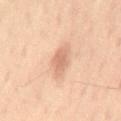Recorded during total-body skin imaging; not selected for excision or biopsy. The tile uses cross-polarized illumination. Automated tile analysis of the lesion measured a lesion color around L≈63 a*≈20 b*≈31 in CIELAB and a normalized border contrast of about 6. The subject is a male in their 60s. The lesion is located on the lower back. A close-up tile cropped from a whole-body skin photograph, about 15 mm across.